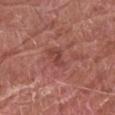Impression:
Part of a total-body skin-imaging series; this lesion was reviewed on a skin check and was not flagged for biopsy.
Context:
A male patient, aged around 80. The lesion is on the right forearm. The tile uses white-light illumination. A 15 mm close-up extracted from a 3D total-body photography capture.